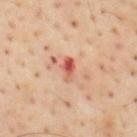Assessment:
The lesion was tiled from a total-body skin photograph and was not biopsied.
Clinical summary:
The lesion is on the mid back. A close-up tile cropped from a whole-body skin photograph, about 15 mm across. A male subject roughly 55 years of age. The recorded lesion diameter is about 2.5 mm.